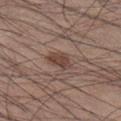Clinical impression:
Imaged during a routine full-body skin examination; the lesion was not biopsied and no histopathology is available.
Clinical summary:
The lesion is located on the right lower leg. A lesion tile, about 15 mm wide, cut from a 3D total-body photograph. An algorithmic analysis of the crop reported a shape eccentricity near 0.8 and a shape-asymmetry score of about 0.25 (0 = symmetric). The lesion's longest dimension is about 3.5 mm. The subject is a male aged 33–37.